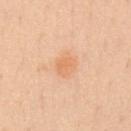Q: Patient demographics?
A: male, approximately 50 years of age
Q: Illumination type?
A: white-light
Q: How was this image acquired?
A: ~15 mm tile from a whole-body skin photo
Q: What is the lesion's diameter?
A: ≈2.5 mm
Q: What is the anatomic site?
A: the chest